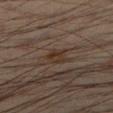follow-up = imaged on a skin check; not biopsied | lesion diameter = ~3 mm (longest diameter) | acquisition = total-body-photography crop, ~15 mm field of view | body site = the right lower leg | tile lighting = cross-polarized | subject = male, about 35 years old.